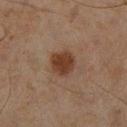follow-up: catalogued during a skin exam; not biopsied | site: the right lower leg | lesion diameter: ≈3 mm | automated metrics: an outline eccentricity of about 0.4 (0 = round, 1 = elongated); a border-irregularity index near 1.5/10, a color-variation rating of about 2/10, and radial color variation of about 0.5 | subject: male, aged approximately 45 | image source: ~15 mm tile from a whole-body skin photo.